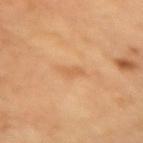workup: no biopsy performed (imaged during a skin exam) | patient: male, aged 58–62 | acquisition: ~15 mm crop, total-body skin-cancer survey | size: about 2.5 mm | site: the right upper arm | automated metrics: a lesion color around L≈61 a*≈22 b*≈40 in CIELAB, about 6 CIELAB-L* units darker than the surrounding skin, and a lesion-to-skin contrast of about 4.5 (normalized; higher = more distinct) | illumination: cross-polarized illumination.